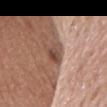Background:
Longest diameter approximately 3 mm. On the head or neck. A 15 mm close-up extracted from a 3D total-body photography capture. The subject is a female aged 63 to 67.